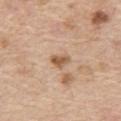On the front of the torso.
A male patient approximately 70 years of age.
About 2.5 mm across.
Imaged with white-light lighting.
A 15 mm close-up tile from a total-body photography series done for melanoma screening.